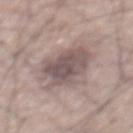Imaged during a routine full-body skin examination; the lesion was not biopsied and no histopathology is available.
Measured at roughly 5.5 mm in maximum diameter.
A male subject, aged around 65.
The lesion-visualizer software estimated an area of roughly 18 mm², an outline eccentricity of about 0.65 (0 = round, 1 = elongated), and two-axis asymmetry of about 0.25. The software also gave a border-irregularity rating of about 3.5/10 and internal color variation of about 4 on a 0–10 scale. The analysis additionally found a detector confidence of about 80 out of 100 that the crop contains a lesion.
On the chest.
A close-up tile cropped from a whole-body skin photograph, about 15 mm across.
This is a white-light tile.